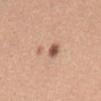Notes:
* follow-up · total-body-photography surveillance lesion; no biopsy
* imaging modality · ~15 mm crop, total-body skin-cancer survey
* lighting · white-light
* patient · female, in their 30s
* body site · the abdomen
* diameter · ~4.5 mm (longest diameter)